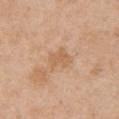workup — catalogued during a skin exam; not biopsied | anatomic site — the chest | subject — female, aged 38–42 | size — ≈3 mm | imaging modality — ~15 mm crop, total-body skin-cancer survey | tile lighting — white-light illumination.